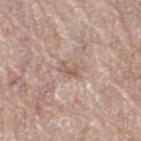Clinical impression:
The lesion was tiled from a total-body skin photograph and was not biopsied.
Image and clinical context:
From the right thigh. The lesion's longest dimension is about 2.5 mm. Captured under white-light illumination. A 15 mm close-up tile from a total-body photography series done for melanoma screening. A female subject, in their 60s.